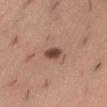| feature | finding |
|---|---|
| biopsy status | total-body-photography surveillance lesion; no biopsy |
| lesion size | about 2.5 mm |
| illumination | white-light |
| acquisition | ~15 mm crop, total-body skin-cancer survey |
| subject | female, about 30 years old |
| anatomic site | the abdomen |